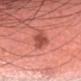Case summary:
• workup — total-body-photography surveillance lesion; no biopsy
• subject — female, aged 43 to 47
• location — the head or neck
• image — ~15 mm tile from a whole-body skin photo